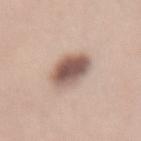The lesion was photographed on a routine skin check and not biopsied; there is no pathology result.
A female patient, aged 33 to 37.
The lesion's longest dimension is about 4 mm.
A 15 mm crop from a total-body photograph taken for skin-cancer surveillance.
Imaged with white-light lighting.
Located on the lower back.